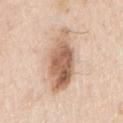<lesion>
  <biopsy_status>not biopsied; imaged during a skin examination</biopsy_status>
  <site>right upper arm</site>
  <image>
    <source>total-body photography crop</source>
    <field_of_view_mm>15</field_of_view_mm>
  </image>
  <patient>
    <sex>male</sex>
    <age_approx>50</age_approx>
  </patient>
  <lesion_size>
    <long_diameter_mm_approx>7.0</long_diameter_mm_approx>
  </lesion_size>
</lesion>